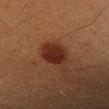Recorded during total-body skin imaging; not selected for excision or biopsy.
A male subject, aged around 40.
Cropped from a total-body skin-imaging series; the visible field is about 15 mm.
Automated image analysis of the tile measured a classifier nevus-likeness of about 100/100 and a lesion-detection confidence of about 100/100.
On the left forearm.
Approximately 3.5 mm at its widest.